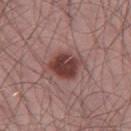{"biopsy_status": "not biopsied; imaged during a skin examination", "automated_metrics": {"shape_asymmetry": 0.2, "cielab_L": 41, "cielab_a": 23, "cielab_b": 21, "vs_skin_darker_L": 14.0, "vs_skin_contrast_norm": 10.5, "border_irregularity_0_10": 2.0, "color_variation_0_10": 4.5, "peripheral_color_asymmetry": 1.5, "nevus_likeness_0_100": 90, "lesion_detection_confidence_0_100": 100}, "lesion_size": {"long_diameter_mm_approx": 4.0}, "patient": {"sex": "male", "age_approx": 55}, "image": {"source": "total-body photography crop", "field_of_view_mm": 15}, "site": "left thigh"}